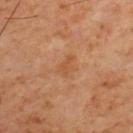Captured during whole-body skin photography for melanoma surveillance; the lesion was not biopsied. Located on the chest. Captured under cross-polarized illumination. Automated tile analysis of the lesion measured a lesion-to-skin contrast of about 5 (normalized; higher = more distinct). And it measured a border-irregularity index near 4/10, a color-variation rating of about 2/10, and peripheral color asymmetry of about 1. Approximately 2.5 mm at its widest. This image is a 15 mm lesion crop taken from a total-body photograph. The subject is a male aged 58–62.